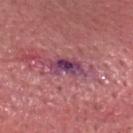The lesion's longest dimension is about 4 mm.
This is a white-light tile.
A male subject aged 73 to 77.
The lesion is on the head or neck.
This image is a 15 mm lesion crop taken from a total-body photograph.